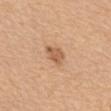{
  "lesion_size": {
    "long_diameter_mm_approx": 2.5
  },
  "lighting": "white-light",
  "patient": {
    "sex": "female",
    "age_approx": 55
  },
  "site": "abdomen",
  "image": {
    "source": "total-body photography crop",
    "field_of_view_mm": 15
  }
}